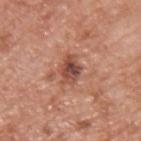{"biopsy_status": "not biopsied; imaged during a skin examination", "image": {"source": "total-body photography crop", "field_of_view_mm": 15}, "patient": {"sex": "male", "age_approx": 60}, "lighting": "white-light", "site": "back"}